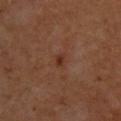The lesion was photographed on a routine skin check and not biopsied; there is no pathology result. The subject is a female aged around 50. Approximately 2 mm at its widest. Automated tile analysis of the lesion measured a color-variation rating of about 1.5/10 and peripheral color asymmetry of about 0.5. It also reported an automated nevus-likeness rating near 15 out of 100. A close-up tile cropped from a whole-body skin photograph, about 15 mm across. Captured under cross-polarized illumination. The lesion is located on the upper back.